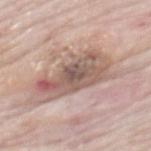No biopsy was performed on this lesion — it was imaged during a full skin examination and was not determined to be concerning.
The subject is a male in their mid- to late 80s or older.
The lesion is on the back.
A region of skin cropped from a whole-body photographic capture, roughly 15 mm wide.
Measured at roughly 7.5 mm in maximum diameter.
Captured under white-light illumination.